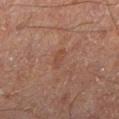workup = no biopsy performed (imaged during a skin exam) | tile lighting = cross-polarized illumination | diameter = about 2.5 mm | subject = male, approximately 65 years of age | image = 15 mm crop, total-body photography | anatomic site = the left lower leg.